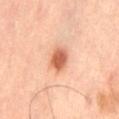Findings:
- workup: total-body-photography surveillance lesion; no biopsy
- site: the left thigh
- diameter: ~3.5 mm (longest diameter)
- image: total-body-photography crop, ~15 mm field of view
- image-analysis metrics: a lesion color around L≈60 a*≈27 b*≈35 in CIELAB and a lesion–skin lightness drop of about 15; a classifier nevus-likeness of about 100/100
- lighting: cross-polarized illumination
- subject: male, aged 63–67